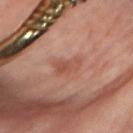Q: What is the imaging modality?
A: 15 mm crop, total-body photography
Q: What are the patient's age and sex?
A: female, in their mid- to late 60s
Q: Where on the body is the lesion?
A: the right forearm